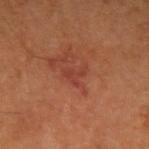Q: Was this lesion biopsied?
A: total-body-photography surveillance lesion; no biopsy
Q: What kind of image is this?
A: ~15 mm tile from a whole-body skin photo
Q: Automated lesion metrics?
A: about 6 CIELAB-L* units darker than the surrounding skin and a normalized lesion–skin contrast near 5
Q: Where on the body is the lesion?
A: the right upper arm
Q: Patient demographics?
A: male, in their mid- to late 60s
Q: Lesion size?
A: ~2.5 mm (longest diameter)
Q: Illumination type?
A: cross-polarized illumination